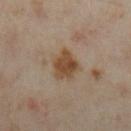workup: total-body-photography surveillance lesion; no biopsy
subject: female, in their mid- to late 30s
anatomic site: the right lower leg
imaging modality: ~15 mm tile from a whole-body skin photo
diameter: ~4 mm (longest diameter)